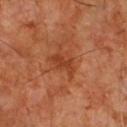patient = male, aged around 60 | site = the chest | image source = ~15 mm crop, total-body skin-cancer survey.